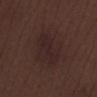{"biopsy_status": "not biopsied; imaged during a skin examination", "automated_metrics": {"cielab_L": 22, "cielab_a": 15, "cielab_b": 15, "border_irregularity_0_10": 2.5, "color_variation_0_10": 1.5, "peripheral_color_asymmetry": 0.5, "nevus_likeness_0_100": 0, "lesion_detection_confidence_0_100": 100}, "patient": {"sex": "male", "age_approx": 70}, "lesion_size": {"long_diameter_mm_approx": 5.0}, "image": {"source": "total-body photography crop", "field_of_view_mm": 15}, "site": "right lower leg", "lighting": "white-light"}